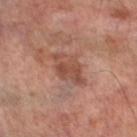Part of a total-body skin-imaging series; this lesion was reviewed on a skin check and was not flagged for biopsy.
Captured under cross-polarized illumination.
A 15 mm crop from a total-body photograph taken for skin-cancer surveillance.
On the left thigh.
Approximately 4.5 mm at its widest.
A male patient roughly 65 years of age.